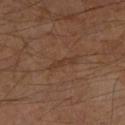No biopsy was performed on this lesion — it was imaged during a full skin examination and was not determined to be concerning. Cropped from a total-body skin-imaging series; the visible field is about 15 mm. This is a cross-polarized tile. Approximately 3.5 mm at its widest. A male patient aged 58 to 62. The lesion is on the left lower leg.